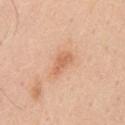| feature | finding |
|---|---|
| workup | total-body-photography surveillance lesion; no biopsy |
| image-analysis metrics | an average lesion color of about L≈64 a*≈25 b*≈35 (CIELAB), about 9 CIELAB-L* units darker than the surrounding skin, and a lesion-to-skin contrast of about 6 (normalized; higher = more distinct); a border-irregularity index near 4/10, a color-variation rating of about 1/10, and a peripheral color-asymmetry measure near 0.5; a detector confidence of about 100 out of 100 that the crop contains a lesion |
| diameter | about 3 mm |
| image | 15 mm crop, total-body photography |
| anatomic site | the chest |
| patient | male, aged approximately 50 |
| illumination | white-light |